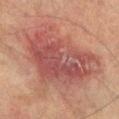<lesion>
<biopsy_status>not biopsied; imaged during a skin examination</biopsy_status>
<image>
  <source>total-body photography crop</source>
  <field_of_view_mm>15</field_of_view_mm>
</image>
<lighting>cross-polarized</lighting>
<patient>
  <sex>male</sex>
  <age_approx>75</age_approx>
</patient>
<site>left lower leg</site>
<automated_metrics>
  <area_mm2_approx>50.0</area_mm2_approx>
  <eccentricity>0.7</eccentricity>
  <border_irregularity_0_10>4.5</border_irregularity_0_10>
</automated_metrics>
<lesion_size>
  <long_diameter_mm_approx>10.0</long_diameter_mm_approx>
</lesion_size>
</lesion>